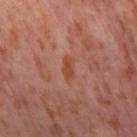Q: What is the imaging modality?
A: ~15 mm tile from a whole-body skin photo
Q: What is the anatomic site?
A: the right thigh
Q: Patient demographics?
A: female, about 55 years old